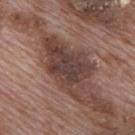Assessment:
The lesion was tiled from a total-body skin photograph and was not biopsied.
Background:
The lesion is located on the mid back. A male patient aged around 70. A 15 mm crop from a total-body photograph taken for skin-cancer surveillance.